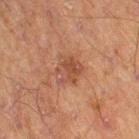Impression:
The lesion was tiled from a total-body skin photograph and was not biopsied.
Context:
A male subject aged approximately 70. A 15 mm close-up extracted from a 3D total-body photography capture. The lesion is on the right thigh. Longest diameter approximately 3 mm.